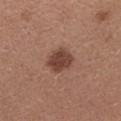  biopsy_status: not biopsied; imaged during a skin examination
  image:
    source: total-body photography crop
    field_of_view_mm: 15
  site: arm
  automated_metrics:
    color_variation_0_10: 2.5
    peripheral_color_asymmetry: 1.0
  patient:
    sex: female
    age_approx: 30
  lighting: white-light
  lesion_size:
    long_diameter_mm_approx: 3.5